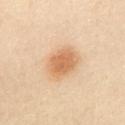{
  "biopsy_status": "not biopsied; imaged during a skin examination",
  "site": "chest",
  "image": {
    "source": "total-body photography crop",
    "field_of_view_mm": 15
  },
  "patient": {
    "sex": "female",
    "age_approx": 40
  }
}